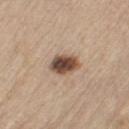Captured during whole-body skin photography for melanoma surveillance; the lesion was not biopsied.
A 15 mm close-up tile from a total-body photography series done for melanoma screening.
The lesion is on the right thigh.
Imaged with white-light lighting.
Measured at roughly 3.5 mm in maximum diameter.
A male subject aged 68–72.